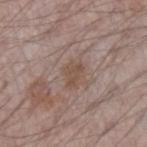From the left forearm. The patient is a male aged approximately 70. Cropped from a whole-body photographic skin survey; the tile spans about 15 mm.